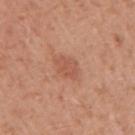Clinical impression: The lesion was tiled from a total-body skin photograph and was not biopsied. Image and clinical context: The subject is a male in their 50s. Approximately 3.5 mm at its widest. The lesion is located on the right upper arm. Cropped from a whole-body photographic skin survey; the tile spans about 15 mm. This is a white-light tile.